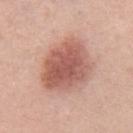Clinical impression: The lesion was photographed on a routine skin check and not biopsied; there is no pathology result. Background: A male patient, aged 53–57. From the chest. Cropped from a total-body skin-imaging series; the visible field is about 15 mm. This is a white-light tile.